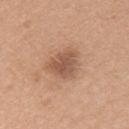follow-up: catalogued during a skin exam; not biopsied
image-analysis metrics: a border-irregularity index near 3.5/10 and a color-variation rating of about 3/10; a classifier nevus-likeness of about 50/100 and a detector confidence of about 100 out of 100 that the crop contains a lesion
diameter: ≈3.5 mm
patient: female, aged around 20
acquisition: ~15 mm crop, total-body skin-cancer survey
illumination: white-light
anatomic site: the left upper arm